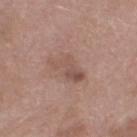<tbp_lesion>
  <biopsy_status>not biopsied; imaged during a skin examination</biopsy_status>
  <image>
    <source>total-body photography crop</source>
    <field_of_view_mm>15</field_of_view_mm>
  </image>
  <patient>
    <sex>female</sex>
    <age_approx>70</age_approx>
  </patient>
  <site>left thigh</site>
  <lesion_size>
    <long_diameter_mm_approx>5.0</long_diameter_mm_approx>
  </lesion_size>
  <lighting>white-light</lighting>
  <automated_metrics>
    <area_mm2_approx>8.0</area_mm2_approx>
    <eccentricity>0.9</eccentricity>
    <shape_asymmetry>0.4</shape_asymmetry>
    <border_irregularity_0_10>5.0</border_irregularity_0_10>
    <color_variation_0_10>4.0</color_variation_0_10>
    <peripheral_color_asymmetry>1.0</peripheral_color_asymmetry>
    <nevus_likeness_0_100>5</nevus_likeness_0_100>
    <lesion_detection_confidence_0_100>100</lesion_detection_confidence_0_100>
  </automated_metrics>
</tbp_lesion>